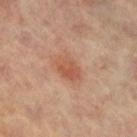The lesion was photographed on a routine skin check and not biopsied; there is no pathology result.
A female subject in their mid- to late 60s.
Located on the leg.
An algorithmic analysis of the crop reported a lesion area of about 6 mm² and a shape eccentricity near 0.7. The analysis additionally found a mean CIELAB color near L≈53 a*≈24 b*≈31, about 8 CIELAB-L* units darker than the surrounding skin, and a lesion-to-skin contrast of about 6.5 (normalized; higher = more distinct). The analysis additionally found a within-lesion color-variation index near 3/10. It also reported a classifier nevus-likeness of about 55/100 and a detector confidence of about 100 out of 100 that the crop contains a lesion.
A 15 mm crop from a total-body photograph taken for skin-cancer surveillance.
Imaged with cross-polarized lighting.